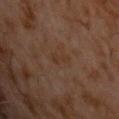Captured during whole-body skin photography for melanoma surveillance; the lesion was not biopsied.
The subject is a male aged approximately 60.
This image is a 15 mm lesion crop taken from a total-body photograph.
The lesion-visualizer software estimated an average lesion color of about L≈31 a*≈16 b*≈25 (CIELAB), roughly 4 lightness units darker than nearby skin, and a normalized border contrast of about 5. The software also gave a border-irregularity index near 5/10, a color-variation rating of about 0/10, and peripheral color asymmetry of about 0. And it measured a lesion-detection confidence of about 100/100.
Captured under cross-polarized illumination.
Located on the chest.
Approximately 2.5 mm at its widest.